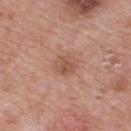Captured during whole-body skin photography for melanoma surveillance; the lesion was not biopsied. On the upper back. A 15 mm crop from a total-body photograph taken for skin-cancer surveillance. The recorded lesion diameter is about 3 mm. A male patient aged around 65.